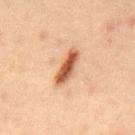From the back. A region of skin cropped from a whole-body photographic capture, roughly 15 mm wide. A male patient about 30 years old.